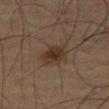Part of a total-body skin-imaging series; this lesion was reviewed on a skin check and was not flagged for biopsy. On the right thigh. Imaged with cross-polarized lighting. A roughly 15 mm field-of-view crop from a total-body skin photograph. The lesion's longest dimension is about 4.5 mm. The subject is a male aged around 65.